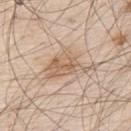<lesion>
  <patient>
    <sex>male</sex>
    <age_approx>80</age_approx>
  </patient>
  <site>upper back</site>
  <image>
    <source>total-body photography crop</source>
    <field_of_view_mm>15</field_of_view_mm>
  </image>
  <lesion_size>
    <long_diameter_mm_approx>6.0</long_diameter_mm_approx>
  </lesion_size>
</lesion>